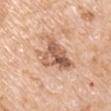Assessment: No biopsy was performed on this lesion — it was imaged during a full skin examination and was not determined to be concerning. Clinical summary: The lesion is on the arm. The subject is a male in their mid- to late 60s. A 15 mm close-up extracted from a 3D total-body photography capture. This is a white-light tile.